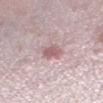{"biopsy_status": "not biopsied; imaged during a skin examination", "lighting": "white-light", "image": {"source": "total-body photography crop", "field_of_view_mm": 15}, "patient": {"sex": "female", "age_approx": 45}, "automated_metrics": {"area_mm2_approx": 4.5, "eccentricity": 0.55, "shape_asymmetry": 0.3, "nevus_likeness_0_100": 5, "lesion_detection_confidence_0_100": 80}, "site": "left lower leg", "lesion_size": {"long_diameter_mm_approx": 2.5}}